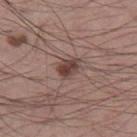Assessment:
This lesion was catalogued during total-body skin photography and was not selected for biopsy.
Background:
Cropped from a whole-body photographic skin survey; the tile spans about 15 mm. Automated tile analysis of the lesion measured a lesion color around L≈42 a*≈18 b*≈21 in CIELAB, roughly 12 lightness units darker than nearby skin, and a lesion-to-skin contrast of about 9.5 (normalized; higher = more distinct). The analysis additionally found border irregularity of about 2 on a 0–10 scale and peripheral color asymmetry of about 1. The software also gave lesion-presence confidence of about 100/100. Located on the leg. Captured under white-light illumination. A male subject in their mid- to late 50s.